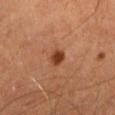Q: Was this lesion biopsied?
A: total-body-photography surveillance lesion; no biopsy
Q: What kind of image is this?
A: 15 mm crop, total-body photography
Q: What are the patient's age and sex?
A: male, aged approximately 65
Q: Where on the body is the lesion?
A: the leg
Q: Illumination type?
A: cross-polarized